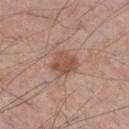size = ~3.5 mm (longest diameter)
lighting = white-light
location = the right thigh
patient = male, aged 53 to 57
acquisition = 15 mm crop, total-body photography